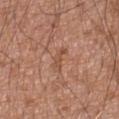Findings:
* automated lesion analysis: a lesion area of about 3.5 mm² and a shape eccentricity near 0.95; a lesion color around L≈51 a*≈23 b*≈31 in CIELAB, about 7 CIELAB-L* units darker than the surrounding skin, and a lesion-to-skin contrast of about 5.5 (normalized; higher = more distinct); internal color variation of about 0 on a 0–10 scale and a peripheral color-asymmetry measure near 0; a nevus-likeness score of about 0/100 and lesion-presence confidence of about 100/100
* illumination: white-light
* diameter: ~3.5 mm (longest diameter)
* subject: male, approximately 75 years of age
* site: the abdomen
* image source: total-body-photography crop, ~15 mm field of view